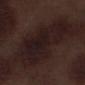  biopsy_status: not biopsied; imaged during a skin examination
  patient:
    sex: male
    age_approx: 70
  site: left lower leg
  image:
    source: total-body photography crop
    field_of_view_mm: 15